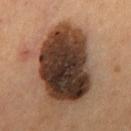Assessment: No biopsy was performed on this lesion — it was imaged during a full skin examination and was not determined to be concerning. Clinical summary: A male subject, aged 53–57. A roughly 15 mm field-of-view crop from a total-body skin photograph. On the abdomen.